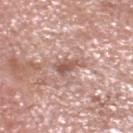Part of a total-body skin-imaging series; this lesion was reviewed on a skin check and was not flagged for biopsy.
Cropped from a whole-body photographic skin survey; the tile spans about 15 mm.
The lesion is located on the head or neck.
A male patient aged 68–72.
This is a white-light tile.
Automated image analysis of the tile measured an area of roughly 4.5 mm², an eccentricity of roughly 0.85, and a symmetry-axis asymmetry near 0.45. The software also gave a normalized lesion–skin contrast near 7.5. It also reported a detector confidence of about 85 out of 100 that the crop contains a lesion.
Measured at roughly 3 mm in maximum diameter.